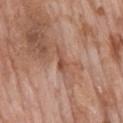Image and clinical context:
The patient is a male in their mid- to late 70s. Longest diameter approximately 3 mm. The tile uses white-light illumination. Automated tile analysis of the lesion measured border irregularity of about 4 on a 0–10 scale, a within-lesion color-variation index near 3/10, and a peripheral color-asymmetry measure near 1. The software also gave a classifier nevus-likeness of about 0/100 and lesion-presence confidence of about 65/100. This image is a 15 mm lesion crop taken from a total-body photograph. On the back.